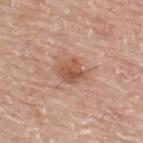The lesion-visualizer software estimated an outline eccentricity of about 0.55 (0 = round, 1 = elongated) and a symmetry-axis asymmetry near 0.2. The software also gave lesion-presence confidence of about 100/100.
The lesion is located on the back.
A close-up tile cropped from a whole-body skin photograph, about 15 mm across.
About 3 mm across.
A male patient roughly 75 years of age.
Imaged with white-light lighting.